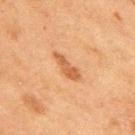Recorded during total-body skin imaging; not selected for excision or biopsy. A close-up tile cropped from a whole-body skin photograph, about 15 mm across. On the mid back. Longest diameter approximately 4 mm. The patient is a male aged approximately 75.